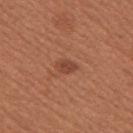follow-up = total-body-photography surveillance lesion; no biopsy
subject = female, in their mid- to late 50s
image = total-body-photography crop, ~15 mm field of view
site = the right upper arm